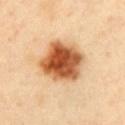• diameter: about 5.5 mm
• acquisition: ~15 mm crop, total-body skin-cancer survey
• TBP lesion metrics: a footprint of about 21 mm², an eccentricity of roughly 0.4, and two-axis asymmetry of about 0.15; a mean CIELAB color near L≈57 a*≈26 b*≈41, a lesion–skin lightness drop of about 21, and a lesion-to-skin contrast of about 13 (normalized; higher = more distinct); a border-irregularity rating of about 1.5/10 and a within-lesion color-variation index near 8/10; a nevus-likeness score of about 100/100 and a lesion-detection confidence of about 100/100
• anatomic site: the chest
• patient: male, aged around 40